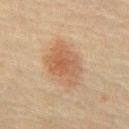• biopsy status: catalogued during a skin exam; not biopsied
• image: total-body-photography crop, ~15 mm field of view
• subject: male, aged approximately 70
• site: the abdomen
• lighting: cross-polarized
• lesion size: about 6 mm